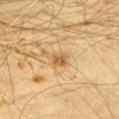The lesion was photographed on a routine skin check and not biopsied; there is no pathology result. A 15 mm close-up tile from a total-body photography series done for melanoma screening. The lesion is located on the chest. A male patient, aged around 70.